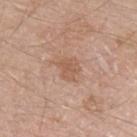The lesion was photographed on a routine skin check and not biopsied; there is no pathology result. Measured at roughly 2.5 mm in maximum diameter. Automated tile analysis of the lesion measured a lesion area of about 5 mm² and a shape-asymmetry score of about 0.3 (0 = symmetric). The lesion is on the arm. A 15 mm close-up extracted from a 3D total-body photography capture. The tile uses white-light illumination. A male subject aged around 60.